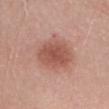follow-up=total-body-photography surveillance lesion; no biopsy | lesion diameter=≈5 mm | site=the head or neck | illumination=white-light | image=~15 mm crop, total-body skin-cancer survey | patient=female, aged 28–32.